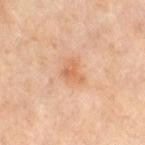Recorded during total-body skin imaging; not selected for excision or biopsy. The lesion-visualizer software estimated an area of roughly 3.5 mm², a shape eccentricity near 0.7, and a symmetry-axis asymmetry near 0.6. It also reported a mean CIELAB color near L≈62 a*≈23 b*≈37, roughly 8 lightness units darker than nearby skin, and a lesion-to-skin contrast of about 6 (normalized; higher = more distinct). And it measured internal color variation of about 1.5 on a 0–10 scale and a peripheral color-asymmetry measure near 0.5. A region of skin cropped from a whole-body photographic capture, roughly 15 mm wide. Imaged with cross-polarized lighting. About 3 mm across. A female patient about 50 years old. From the right thigh.